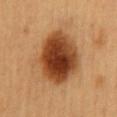{"biopsy_status": "not biopsied; imaged during a skin examination", "lighting": "cross-polarized", "patient": {"sex": "female", "age_approx": 60}, "automated_metrics": {"area_mm2_approx": 30.0, "eccentricity": 0.65, "cielab_L": 45, "cielab_a": 26, "cielab_b": 39, "vs_skin_contrast_norm": 13.0, "border_irregularity_0_10": 1.5, "peripheral_color_asymmetry": 2.5, "nevus_likeness_0_100": 100, "lesion_detection_confidence_0_100": 100}, "image": {"source": "total-body photography crop", "field_of_view_mm": 15}, "site": "mid back", "lesion_size": {"long_diameter_mm_approx": 7.0}}